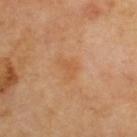No biopsy was performed on this lesion — it was imaged during a full skin examination and was not determined to be concerning.
On the upper back.
This image is a 15 mm lesion crop taken from a total-body photograph.